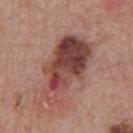Case summary:
– follow-up — imaged on a skin check; not biopsied
– image-analysis metrics — an area of roughly 27 mm² and a shape eccentricity near 0.85
– subject — male, roughly 65 years of age
– lesion diameter — ~8.5 mm (longest diameter)
– lighting — white-light illumination
– anatomic site — the chest
– imaging modality — ~15 mm crop, total-body skin-cancer survey